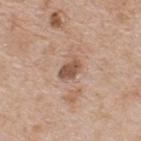Measured at roughly 2.5 mm in maximum diameter. The lesion-visualizer software estimated a mean CIELAB color near L≈52 a*≈19 b*≈28, about 13 CIELAB-L* units darker than the surrounding skin, and a normalized border contrast of about 8.5. The software also gave a classifier nevus-likeness of about 15/100 and a detector confidence of about 100 out of 100 that the crop contains a lesion. A 15 mm close-up extracted from a 3D total-body photography capture. A male patient aged approximately 65. The tile uses white-light illumination. The lesion is located on the upper back.